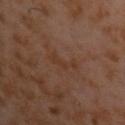workup: total-body-photography surveillance lesion; no biopsy | tile lighting: cross-polarized | automated lesion analysis: a lesion color around L≈32 a*≈17 b*≈26 in CIELAB, roughly 4 lightness units darker than nearby skin, and a normalized lesion–skin contrast near 5.5; a lesion-detection confidence of about 100/100 | size: ≈4 mm | acquisition: total-body-photography crop, ~15 mm field of view | location: the chest | patient: male, about 60 years old.